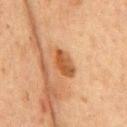No biopsy was performed on this lesion — it was imaged during a full skin examination and was not determined to be concerning.
A male subject aged around 75.
From the front of the torso.
A roughly 15 mm field-of-view crop from a total-body skin photograph.